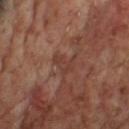<lesion>
  <biopsy_status>not biopsied; imaged during a skin examination</biopsy_status>
  <image>
    <source>total-body photography crop</source>
    <field_of_view_mm>15</field_of_view_mm>
  </image>
  <lesion_size>
    <long_diameter_mm_approx>2.5</long_diameter_mm_approx>
  </lesion_size>
  <lighting>cross-polarized</lighting>
  <site>chest</site>
  <patient>
    <sex>male</sex>
    <age_approx>65</age_approx>
  </patient>
</lesion>